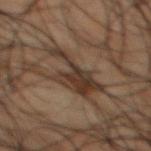Q: Was a biopsy performed?
A: total-body-photography surveillance lesion; no biopsy
Q: What did automated image analysis measure?
A: a footprint of about 13 mm² and a symmetry-axis asymmetry near 0.5; a lesion color around L≈26 a*≈11 b*≈19 in CIELAB and a normalized lesion–skin contrast near 9; a lesion-detection confidence of about 65/100
Q: What are the patient's age and sex?
A: male, approximately 50 years of age
Q: What is the imaging modality?
A: ~15 mm crop, total-body skin-cancer survey
Q: What is the anatomic site?
A: the mid back
Q: Lesion size?
A: about 5.5 mm
Q: What lighting was used for the tile?
A: cross-polarized